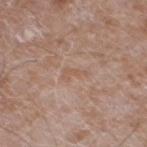Q: Was this lesion biopsied?
A: total-body-photography surveillance lesion; no biopsy
Q: Lesion location?
A: the left lower leg
Q: How large is the lesion?
A: ~2.5 mm (longest diameter)
Q: How was this image acquired?
A: ~15 mm tile from a whole-body skin photo
Q: What did automated image analysis measure?
A: a lesion area of about 3 mm², a shape eccentricity near 0.9, and a shape-asymmetry score of about 0.55 (0 = symmetric); a lesion color around L≈56 a*≈18 b*≈28 in CIELAB, a lesion–skin lightness drop of about 5, and a lesion-to-skin contrast of about 4.5 (normalized; higher = more distinct); a border-irregularity index near 6/10
Q: Who is the patient?
A: male, aged approximately 60